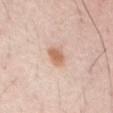Q: Was this lesion biopsied?
A: catalogued during a skin exam; not biopsied
Q: Patient demographics?
A: in their mid-50s
Q: What is the lesion's diameter?
A: about 2.5 mm
Q: Lesion location?
A: the abdomen
Q: What is the imaging modality?
A: 15 mm crop, total-body photography
Q: What did automated image analysis measure?
A: an average lesion color of about L≈62 a*≈20 b*≈30 (CIELAB), roughly 11 lightness units darker than nearby skin, and a normalized lesion–skin contrast near 8